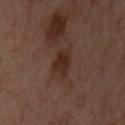Located on the left upper arm.
This image is a 15 mm lesion crop taken from a total-body photograph.
A female subject, about 55 years old.